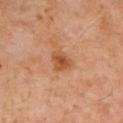Captured during whole-body skin photography for melanoma surveillance; the lesion was not biopsied. A male subject, about 55 years old. On the left upper arm. A 15 mm close-up extracted from a 3D total-body photography capture. The total-body-photography lesion software estimated a footprint of about 4.5 mm², an eccentricity of roughly 0.65, and a shape-asymmetry score of about 0.3 (0 = symmetric). The analysis additionally found an average lesion color of about L≈53 a*≈26 b*≈38 (CIELAB), about 10 CIELAB-L* units darker than the surrounding skin, and a normalized lesion–skin contrast near 7.5.